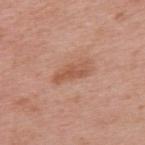Q: Lesion location?
A: the upper back
Q: What lighting was used for the tile?
A: white-light
Q: What is the imaging modality?
A: ~15 mm tile from a whole-body skin photo
Q: Who is the patient?
A: female, aged 38–42
Q: Lesion size?
A: ≈4.5 mm